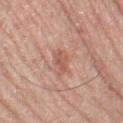Recorded during total-body skin imaging; not selected for excision or biopsy. The recorded lesion diameter is about 2.5 mm. The lesion is on the left thigh. A male patient, aged approximately 70. A 15 mm crop from a total-body photograph taken for skin-cancer surveillance. Imaged with white-light lighting.